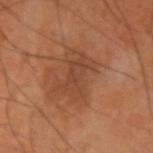Q: Was this lesion biopsied?
A: total-body-photography surveillance lesion; no biopsy
Q: Patient demographics?
A: male, aged 58 to 62
Q: Where on the body is the lesion?
A: the left upper arm
Q: What is the lesion's diameter?
A: ~4 mm (longest diameter)
Q: What is the imaging modality?
A: 15 mm crop, total-body photography
Q: How was the tile lit?
A: cross-polarized illumination